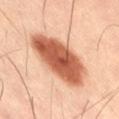TBP lesion metrics: an average lesion color of about L≈57 a*≈28 b*≈35 (CIELAB), roughly 20 lightness units darker than nearby skin, and a normalized lesion–skin contrast near 12
subject: male, roughly 40 years of age
site: the lower back
image: total-body-photography crop, ~15 mm field of view
diameter: about 7.5 mm
illumination: cross-polarized illumination